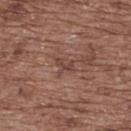- biopsy status: catalogued during a skin exam; not biopsied
- anatomic site: the upper back
- illumination: white-light
- diameter: ≈3 mm
- patient: female, aged 73 to 77
- imaging modality: 15 mm crop, total-body photography
- image-analysis metrics: a border-irregularity rating of about 4.5/10, a within-lesion color-variation index near 2/10, and a peripheral color-asymmetry measure near 0.5; an automated nevus-likeness rating near 0 out of 100 and a detector confidence of about 90 out of 100 that the crop contains a lesion